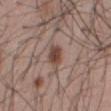The lesion was tiled from a total-body skin photograph and was not biopsied. This is a white-light tile. An algorithmic analysis of the crop reported a shape eccentricity near 0.8 and a shape-asymmetry score of about 0.2 (0 = symmetric). And it measured a nevus-likeness score of about 95/100. The patient is a male about 55 years old. Approximately 3 mm at its widest. The lesion is on the abdomen. Cropped from a whole-body photographic skin survey; the tile spans about 15 mm.